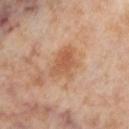This lesion was catalogued during total-body skin photography and was not selected for biopsy. Measured at roughly 4.5 mm in maximum diameter. A female patient about 55 years old. A 15 mm crop from a total-body photograph taken for skin-cancer surveillance. The lesion is located on the left lower leg. Imaged with cross-polarized lighting.